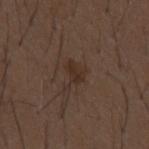The lesion was photographed on a routine skin check and not biopsied; there is no pathology result. On the mid back. A 15 mm crop from a total-body photograph taken for skin-cancer surveillance. The lesion-visualizer software estimated a lesion area of about 5.5 mm², an outline eccentricity of about 0.75 (0 = round, 1 = elongated), and a symmetry-axis asymmetry near 0.6. The analysis additionally found a border-irregularity rating of about 6.5/10, a within-lesion color-variation index near 1.5/10, and peripheral color asymmetry of about 0.5. The software also gave a classifier nevus-likeness of about 0/100 and a detector confidence of about 100 out of 100 that the crop contains a lesion. The subject is a male approximately 50 years of age. Measured at roughly 3.5 mm in maximum diameter.